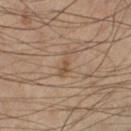No biopsy was performed on this lesion — it was imaged during a full skin examination and was not determined to be concerning.